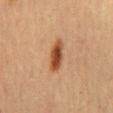This lesion was catalogued during total-body skin photography and was not selected for biopsy. The lesion is on the mid back. The subject is a female aged around 60. The tile uses cross-polarized illumination. An algorithmic analysis of the crop reported a mean CIELAB color near L≈44 a*≈22 b*≈33, roughly 13 lightness units darker than nearby skin, and a lesion-to-skin contrast of about 10 (normalized; higher = more distinct). The lesion's longest dimension is about 4.5 mm. A lesion tile, about 15 mm wide, cut from a 3D total-body photograph.